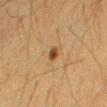biopsy status=total-body-photography surveillance lesion; no biopsy
patient=male, aged 33 to 37
illumination=cross-polarized
size=~2 mm (longest diameter)
image=total-body-photography crop, ~15 mm field of view
anatomic site=the mid back